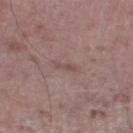| feature | finding |
|---|---|
| biopsy status | total-body-photography surveillance lesion; no biopsy |
| lesion size | ≈2.5 mm |
| subject | male, aged around 50 |
| site | the leg |
| image source | total-body-photography crop, ~15 mm field of view |